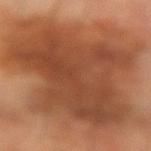The lesion was photographed on a routine skin check and not biopsied; there is no pathology result. A male patient aged around 70. This is a cross-polarized tile. Located on the left forearm. Longest diameter approximately 12 mm. A 15 mm crop from a total-body photograph taken for skin-cancer surveillance.